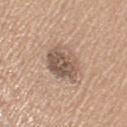Part of a total-body skin-imaging series; this lesion was reviewed on a skin check and was not flagged for biopsy. The total-body-photography lesion software estimated a lesion color around L≈55 a*≈15 b*≈26 in CIELAB, roughly 12 lightness units darker than nearby skin, and a normalized border contrast of about 8. The analysis additionally found a nevus-likeness score of about 10/100 and a detector confidence of about 100 out of 100 that the crop contains a lesion. The lesion is located on the arm. Imaged with white-light lighting. A female patient, in their mid- to late 60s. A close-up tile cropped from a whole-body skin photograph, about 15 mm across. Approximately 4.5 mm at its widest.